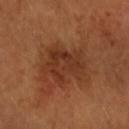A female subject roughly 55 years of age. From the right forearm. A region of skin cropped from a whole-body photographic capture, roughly 15 mm wide. About 5.5 mm across. The total-body-photography lesion software estimated an area of roughly 18 mm² and two-axis asymmetry of about 0.35. The analysis additionally found an average lesion color of about L≈36 a*≈25 b*≈32 (CIELAB) and roughly 8 lightness units darker than nearby skin. The software also gave a border-irregularity index near 4.5/10 and internal color variation of about 3.5 on a 0–10 scale. The software also gave a detector confidence of about 100 out of 100 that the crop contains a lesion.